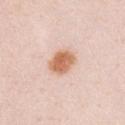<tbp_lesion>
  <biopsy_status>not biopsied; imaged during a skin examination</biopsy_status>
  <site>chest</site>
  <lighting>white-light</lighting>
  <image>
    <source>total-body photography crop</source>
    <field_of_view_mm>15</field_of_view_mm>
  </image>
  <lesion_size>
    <long_diameter_mm_approx>3.5</long_diameter_mm_approx>
  </lesion_size>
  <patient>
    <sex>female</sex>
    <age_approx>30</age_approx>
  </patient>
</tbp_lesion>